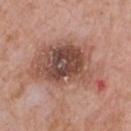Notes:
• biopsy status: no biopsy performed (imaged during a skin exam)
• tile lighting: white-light illumination
• imaging modality: 15 mm crop, total-body photography
• lesion diameter: about 9 mm
• anatomic site: the chest
• TBP lesion metrics: a lesion color around L≈50 a*≈22 b*≈26 in CIELAB, roughly 13 lightness units darker than nearby skin, and a normalized lesion–skin contrast near 9
• patient: male, approximately 60 years of age